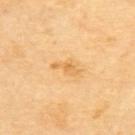Imaged during a routine full-body skin examination; the lesion was not biopsied and no histopathology is available. A lesion tile, about 15 mm wide, cut from a 3D total-body photograph. The lesion's longest dimension is about 3.5 mm. The tile uses cross-polarized illumination. The lesion is located on the upper back. A female subject in their 70s.